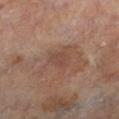Imaged during a routine full-body skin examination; the lesion was not biopsied and no histopathology is available. Longest diameter approximately 2.5 mm. The tile uses cross-polarized illumination. A 15 mm close-up tile from a total-body photography series done for melanoma screening. A male subject roughly 65 years of age. Automated image analysis of the tile measured a lesion area of about 4 mm², an outline eccentricity of about 0.65 (0 = round, 1 = elongated), and two-axis asymmetry of about 0.3. The analysis additionally found lesion-presence confidence of about 100/100. From the left lower leg.